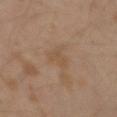Clinical impression: The lesion was tiled from a total-body skin photograph and was not biopsied. Image and clinical context: Located on the left upper arm. Captured under cross-polarized illumination. Cropped from a whole-body photographic skin survey; the tile spans about 15 mm. A male subject approximately 30 years of age.